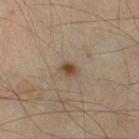patient:
  sex: male
  age_approx: 55
image:
  source: total-body photography crop
  field_of_view_mm: 15
site: right lower leg
lesion_size:
  long_diameter_mm_approx: 2.0
automated_metrics:
  area_mm2_approx: 2.5
  eccentricity: 0.7
  vs_skin_darker_L: 11.0
  color_variation_0_10: 2.0
  nevus_likeness_0_100: 95
  lesion_detection_confidence_0_100: 100